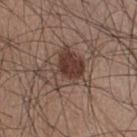Clinical impression: No biopsy was performed on this lesion — it was imaged during a full skin examination and was not determined to be concerning. Acquisition and patient details: A male patient, aged 33–37. A roughly 15 mm field-of-view crop from a total-body skin photograph. The lesion is on the right thigh.